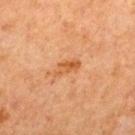Captured during whole-body skin photography for melanoma surveillance; the lesion was not biopsied.
On the mid back.
The tile uses cross-polarized illumination.
Automated image analysis of the tile measured an area of roughly 3.5 mm² and a shape eccentricity near 0.9. The software also gave a classifier nevus-likeness of about 10/100 and a lesion-detection confidence of about 100/100.
About 3 mm across.
Cropped from a total-body skin-imaging series; the visible field is about 15 mm.
A male subject, aged 63 to 67.